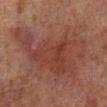Captured during whole-body skin photography for melanoma surveillance; the lesion was not biopsied. A lesion tile, about 15 mm wide, cut from a 3D total-body photograph. The tile uses cross-polarized illumination. The patient is a male aged 68–72. Measured at roughly 11.5 mm in maximum diameter. The lesion is located on the left lower leg.